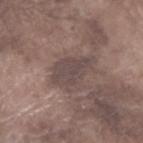<case>
<biopsy_status>not biopsied; imaged during a skin examination</biopsy_status>
<automated_metrics>
  <nevus_likeness_0_100>0</nevus_likeness_0_100>
  <lesion_detection_confidence_0_100>50</lesion_detection_confidence_0_100>
</automated_metrics>
<site>left forearm</site>
<image>
  <source>total-body photography crop</source>
  <field_of_view_mm>15</field_of_view_mm>
</image>
<lighting>white-light</lighting>
<patient>
  <sex>male</sex>
  <age_approx>75</age_approx>
</patient>
</case>